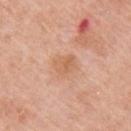Imaged during a routine full-body skin examination; the lesion was not biopsied and no histopathology is available.
Captured under white-light illumination.
A male patient aged 63 to 67.
The lesion is on the right upper arm.
The recorded lesion diameter is about 3 mm.
Cropped from a total-body skin-imaging series; the visible field is about 15 mm.